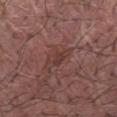{"biopsy_status": "not biopsied; imaged during a skin examination", "site": "left forearm", "lighting": "white-light", "automated_metrics": {"area_mm2_approx": 4.5, "eccentricity": 0.95, "shape_asymmetry": 0.2, "cielab_L": 37, "cielab_a": 21, "cielab_b": 21, "vs_skin_contrast_norm": 5.5, "nevus_likeness_0_100": 0, "lesion_detection_confidence_0_100": 95}, "patient": {"sex": "male", "age_approx": 70}, "image": {"source": "total-body photography crop", "field_of_view_mm": 15}}